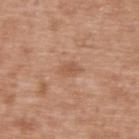biopsy status: total-body-photography surveillance lesion; no biopsy
location: the upper back
lesion diameter: ~2.5 mm (longest diameter)
subject: male, aged approximately 50
acquisition: ~15 mm crop, total-body skin-cancer survey
automated lesion analysis: a lesion area of about 3 mm², an outline eccentricity of about 0.8 (0 = round, 1 = elongated), and a shape-asymmetry score of about 0.25 (0 = symmetric); a mean CIELAB color near L≈55 a*≈21 b*≈33, a lesion–skin lightness drop of about 7, and a normalized border contrast of about 5.5; lesion-presence confidence of about 100/100
tile lighting: white-light